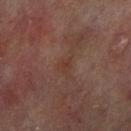No biopsy was performed on this lesion — it was imaged during a full skin examination and was not determined to be concerning. A 15 mm close-up tile from a total-body photography series done for melanoma screening. Imaged with cross-polarized lighting. The total-body-photography lesion software estimated a lesion area of about 1.5 mm² and a symmetry-axis asymmetry near 0.3. It also reported a mean CIELAB color near L≈35 a*≈20 b*≈24 and a normalized lesion–skin contrast near 5. And it measured an automated nevus-likeness rating near 0 out of 100 and a detector confidence of about 100 out of 100 that the crop contains a lesion. On the right lower leg. A male patient, roughly 70 years of age.